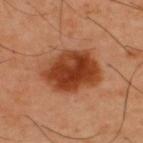Clinical impression: Part of a total-body skin-imaging series; this lesion was reviewed on a skin check and was not flagged for biopsy. Context: The lesion is on the upper back. About 6.5 mm across. An algorithmic analysis of the crop reported a lesion area of about 25 mm², an eccentricity of roughly 0.7, and a symmetry-axis asymmetry near 0.15. A 15 mm close-up extracted from a 3D total-body photography capture. This is a cross-polarized tile. A male patient aged 38 to 42.